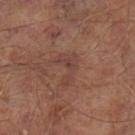Notes:
• follow-up: imaged on a skin check; not biopsied
• image: ~15 mm tile from a whole-body skin photo
• patient: male, aged approximately 65
• location: the left lower leg
• size: about 3.5 mm
• TBP lesion metrics: a symmetry-axis asymmetry near 0.7; a border-irregularity index near 8.5/10, a color-variation rating of about 2/10, and peripheral color asymmetry of about 0.5; a classifier nevus-likeness of about 0/100 and lesion-presence confidence of about 100/100